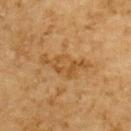| feature | finding |
|---|---|
| follow-up | catalogued during a skin exam; not biopsied |
| subject | male, aged 83 or over |
| imaging modality | ~15 mm crop, total-body skin-cancer survey |
| lesion size | ≈6.5 mm |
| tile lighting | cross-polarized illumination |
| site | the upper back |